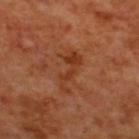Imaged during a routine full-body skin examination; the lesion was not biopsied and no histopathology is available.
Located on the upper back.
A region of skin cropped from a whole-body photographic capture, roughly 15 mm wide.
Measured at roughly 4.5 mm in maximum diameter.
This is a cross-polarized tile.
The patient is a male approximately 70 years of age.